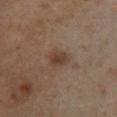Impression:
Imaged during a routine full-body skin examination; the lesion was not biopsied and no histopathology is available.
Image and clinical context:
A male patient, aged 63–67. A 15 mm crop from a total-body photograph taken for skin-cancer surveillance. The lesion is on the left lower leg. Approximately 2.5 mm at its widest.